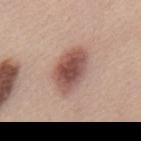| field | value |
|---|---|
| site | the mid back |
| subject | male, aged approximately 45 |
| imaging modality | ~15 mm tile from a whole-body skin photo |